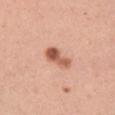This lesion was catalogued during total-body skin photography and was not selected for biopsy. A roughly 15 mm field-of-view crop from a total-body skin photograph. The recorded lesion diameter is about 4 mm. Located on the left thigh. The patient is a female aged approximately 30. Imaged with white-light lighting. The total-body-photography lesion software estimated an automated nevus-likeness rating near 100 out of 100.